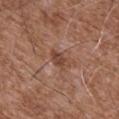The lesion was photographed on a routine skin check and not biopsied; there is no pathology result. Located on the chest. A male patient aged 73 to 77. Longest diameter approximately 3 mm. A 15 mm close-up extracted from a 3D total-body photography capture. Imaged with white-light lighting.